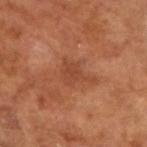Case summary:
– follow-up — no biopsy performed (imaged during a skin exam)
– patient — male, about 65 years old
– lighting — cross-polarized
– TBP lesion metrics — a border-irregularity rating of about 6/10, internal color variation of about 2 on a 0–10 scale, and a peripheral color-asymmetry measure near 0.5
– imaging modality — ~15 mm crop, total-body skin-cancer survey